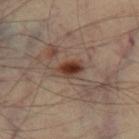* biopsy status — total-body-photography surveillance lesion; no biopsy